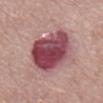Case summary:
- biopsy status: no biopsy performed (imaged during a skin exam)
- acquisition: 15 mm crop, total-body photography
- image-analysis metrics: a border-irregularity index near 2/10; an automated nevus-likeness rating near 70 out of 100 and a lesion-detection confidence of about 100/100
- body site: the abdomen
- patient: male, in their mid- to late 60s
- lighting: white-light
- lesion diameter: ~6 mm (longest diameter)